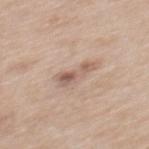notes: no biopsy performed (imaged during a skin exam) | illumination: white-light | acquisition: ~15 mm crop, total-body skin-cancer survey | patient: female, roughly 40 years of age | location: the mid back | lesion size: about 4 mm.